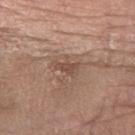follow-up: no biopsy performed (imaged during a skin exam) | image: total-body-photography crop, ~15 mm field of view | patient: female, in their mid-70s | illumination: white-light illumination | lesion diameter: about 2.5 mm | body site: the arm | automated metrics: about 9 CIELAB-L* units darker than the surrounding skin and a normalized border contrast of about 6.5; internal color variation of about 0.5 on a 0–10 scale and a peripheral color-asymmetry measure near 0; a classifier nevus-likeness of about 5/100 and a lesion-detection confidence of about 100/100.